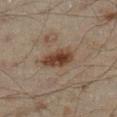Assessment: Captured during whole-body skin photography for melanoma surveillance; the lesion was not biopsied. Acquisition and patient details: The patient is a male in their mid- to late 40s. A close-up tile cropped from a whole-body skin photograph, about 15 mm across. The lesion is on the left lower leg.